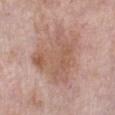– follow-up — catalogued during a skin exam; not biopsied
– imaging modality — ~15 mm crop, total-body skin-cancer survey
– diameter — ~7 mm (longest diameter)
– site — the left lower leg
– subject — female, aged around 60
– tile lighting — white-light illumination